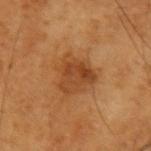Impression:
The lesion was tiled from a total-body skin photograph and was not biopsied.
Clinical summary:
The tile uses cross-polarized illumination. The lesion is on the right upper arm. A male subject, in their 60s. A 15 mm close-up extracted from a 3D total-body photography capture. Longest diameter approximately 4.5 mm.